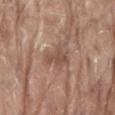| key | value |
|---|---|
| workup | imaged on a skin check; not biopsied |
| image-analysis metrics | two-axis asymmetry of about 0.4; a border-irregularity rating of about 4.5/10, a within-lesion color-variation index near 1.5/10, and peripheral color asymmetry of about 0.5 |
| site | the back |
| lesion diameter | about 3.5 mm |
| illumination | white-light illumination |
| subject | male, aged approximately 80 |
| imaging modality | 15 mm crop, total-body photography |